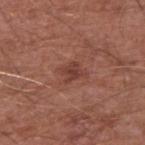Captured during whole-body skin photography for melanoma surveillance; the lesion was not biopsied.
A region of skin cropped from a whole-body photographic capture, roughly 15 mm wide.
From the right upper arm.
The patient is a male aged 63–67.
Imaged with white-light lighting.
Longest diameter approximately 3 mm.